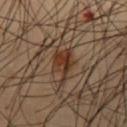Q: Is there a histopathology result?
A: no biopsy performed (imaged during a skin exam)
Q: Patient demographics?
A: male, roughly 55 years of age
Q: What is the anatomic site?
A: the abdomen
Q: Illumination type?
A: cross-polarized
Q: What kind of image is this?
A: 15 mm crop, total-body photography